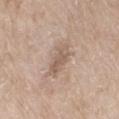{
  "biopsy_status": "not biopsied; imaged during a skin examination",
  "patient": {
    "sex": "female",
    "age_approx": 75
  },
  "image": {
    "source": "total-body photography crop",
    "field_of_view_mm": 15
  },
  "lesion_size": {
    "long_diameter_mm_approx": 4.5
  },
  "site": "leg",
  "automated_metrics": {
    "area_mm2_approx": 7.5,
    "eccentricity": 0.9,
    "shape_asymmetry": 0.25,
    "vs_skin_darker_L": 9.0,
    "vs_skin_contrast_norm": 6.0,
    "peripheral_color_asymmetry": 1.0
  }
}